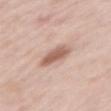Part of a total-body skin-imaging series; this lesion was reviewed on a skin check and was not flagged for biopsy.
Imaged with white-light lighting.
From the left upper arm.
Cropped from a total-body skin-imaging series; the visible field is about 15 mm.
The patient is a female aged approximately 50.